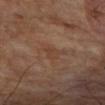Impression: This lesion was catalogued during total-body skin photography and was not selected for biopsy. Acquisition and patient details: A male subject aged 68–72. From the arm. A roughly 15 mm field-of-view crop from a total-body skin photograph.